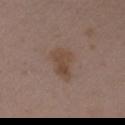Captured during whole-body skin photography for melanoma surveillance; the lesion was not biopsied. A lesion tile, about 15 mm wide, cut from a 3D total-body photograph. The tile uses white-light illumination. A female patient roughly 35 years of age. The lesion's longest dimension is about 4 mm. The lesion is on the right upper arm.